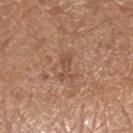biopsy status = catalogued during a skin exam; not biopsied
size = ~3.5 mm (longest diameter)
location = the left upper arm
automated metrics = an average lesion color of about L≈50 a*≈20 b*≈30 (CIELAB) and a normalized border contrast of about 5.5
lighting = white-light
patient = male, aged around 70
image = 15 mm crop, total-body photography